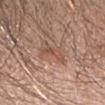Q: Was this lesion biopsied?
A: catalogued during a skin exam; not biopsied
Q: Who is the patient?
A: female, about 40 years old
Q: What is the imaging modality?
A: total-body-photography crop, ~15 mm field of view
Q: How was the tile lit?
A: white-light illumination
Q: What is the anatomic site?
A: the head or neck
Q: Automated lesion metrics?
A: a lesion area of about 4.5 mm² and an outline eccentricity of about 0.9 (0 = round, 1 = elongated); an average lesion color of about L≈51 a*≈20 b*≈28 (CIELAB) and roughly 7 lightness units darker than nearby skin; a lesion-detection confidence of about 100/100